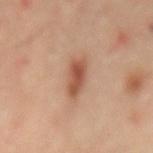Case summary:
- biopsy status · catalogued during a skin exam; not biopsied
- anatomic site · the lower back
- illumination · cross-polarized illumination
- subject · male, in their mid-60s
- diameter · ≈3.5 mm
- image · total-body-photography crop, ~15 mm field of view
- image-analysis metrics · a mean CIELAB color near L≈51 a*≈23 b*≈31 and a normalized border contrast of about 8.5; a border-irregularity index near 2/10 and a color-variation rating of about 3.5/10